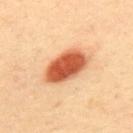| feature | finding |
|---|---|
| biopsy status | catalogued during a skin exam; not biopsied |
| patient | male, aged approximately 40 |
| anatomic site | the upper back |
| image source | ~15 mm crop, total-body skin-cancer survey |